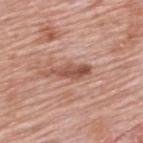Q: Was this lesion biopsied?
A: total-body-photography surveillance lesion; no biopsy
Q: What is the lesion's diameter?
A: about 5 mm
Q: Who is the patient?
A: male, aged 78–82
Q: What lighting was used for the tile?
A: white-light illumination
Q: What is the imaging modality?
A: total-body-photography crop, ~15 mm field of view
Q: Where on the body is the lesion?
A: the upper back